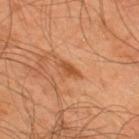Imaged during a routine full-body skin examination; the lesion was not biopsied and no histopathology is available. A roughly 15 mm field-of-view crop from a total-body skin photograph. A male patient, approximately 45 years of age. From the upper back.